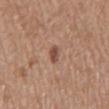Notes:
* workup · catalogued during a skin exam; not biopsied
* anatomic site · the mid back
* imaging modality · total-body-photography crop, ~15 mm field of view
* patient · male, in their 70s